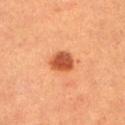workup: total-body-photography surveillance lesion; no biopsy
lighting: cross-polarized
size: ~3 mm (longest diameter)
image-analysis metrics: an area of roughly 6 mm², a shape eccentricity near 0.7, and a shape-asymmetry score of about 0.2 (0 = symmetric); a border-irregularity index near 2/10, internal color variation of about 4 on a 0–10 scale, and a peripheral color-asymmetry measure near 1.5; a classifier nevus-likeness of about 100/100
site: the left lower leg
imaging modality: total-body-photography crop, ~15 mm field of view
subject: female, aged 53–57